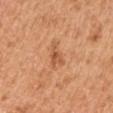Findings:
– workup · total-body-photography surveillance lesion; no biopsy
– automated lesion analysis · an area of roughly 4.5 mm² and two-axis asymmetry of about 0.45; an average lesion color of about L≈57 a*≈26 b*≈38 (CIELAB), roughly 9 lightness units darker than nearby skin, and a normalized lesion–skin contrast near 6; a detector confidence of about 100 out of 100 that the crop contains a lesion
– lighting · white-light
– patient · male, aged 53–57
– image source · total-body-photography crop, ~15 mm field of view
– lesion diameter · ~3.5 mm (longest diameter)
– body site · the right upper arm